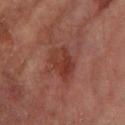Q: Was this lesion biopsied?
A: catalogued during a skin exam; not biopsied
Q: Who is the patient?
A: male, roughly 85 years of age or older
Q: Lesion location?
A: the left forearm
Q: Illumination type?
A: cross-polarized
Q: What is the imaging modality?
A: total-body-photography crop, ~15 mm field of view
Q: What did automated image analysis measure?
A: a footprint of about 9 mm² and a shape-asymmetry score of about 0.2 (0 = symmetric); an automated nevus-likeness rating near 40 out of 100 and lesion-presence confidence of about 100/100